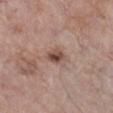Captured during whole-body skin photography for melanoma surveillance; the lesion was not biopsied. The subject is a female aged 68–72. On the right lower leg. About 2.5 mm across. This is a white-light tile. A 15 mm crop from a total-body photograph taken for skin-cancer surveillance.